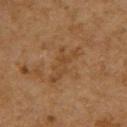Case summary:
* workup: catalogued during a skin exam; not biopsied
* location: the upper back
* patient: female, aged 58–62
* illumination: cross-polarized
* image: ~15 mm crop, total-body skin-cancer survey
* automated lesion analysis: a footprint of about 8.5 mm², a shape eccentricity near 0.9, and two-axis asymmetry of about 0.55; an automated nevus-likeness rating near 0 out of 100